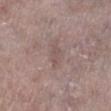biopsy_status: not biopsied; imaged during a skin examination
image:
  source: total-body photography crop
  field_of_view_mm: 15
lesion_size:
  long_diameter_mm_approx: 2.5
lighting: white-light
site: left lower leg
patient:
  sex: female
  age_approx: 65